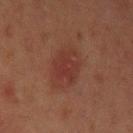Impression: This lesion was catalogued during total-body skin photography and was not selected for biopsy. Clinical summary: A male subject about 45 years old. Longest diameter approximately 4 mm. A 15 mm close-up tile from a total-body photography series done for melanoma screening. On the left upper arm.